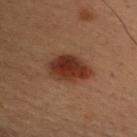Recorded during total-body skin imaging; not selected for excision or biopsy.
The lesion is on the chest.
A male patient about 30 years old.
This is a cross-polarized tile.
A 15 mm close-up tile from a total-body photography series done for melanoma screening.